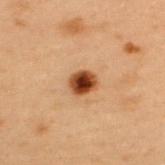Impression:
Recorded during total-body skin imaging; not selected for excision or biopsy.
Image and clinical context:
A region of skin cropped from a whole-body photographic capture, roughly 15 mm wide. Located on the back. The patient is a male about 55 years old.